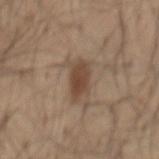A male subject, aged approximately 55.
The recorded lesion diameter is about 4 mm.
Captured under white-light illumination.
Cropped from a total-body skin-imaging series; the visible field is about 15 mm.
On the mid back.